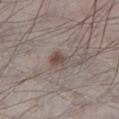The lesion was photographed on a routine skin check and not biopsied; there is no pathology result. An algorithmic analysis of the crop reported an area of roughly 4 mm² and an eccentricity of roughly 0.65. And it measured an automated nevus-likeness rating near 90 out of 100 and lesion-presence confidence of about 100/100. Imaged with white-light lighting. Approximately 3 mm at its widest. The patient is a male aged 68–72. A 15 mm close-up extracted from a 3D total-body photography capture. On the left lower leg.